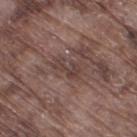Captured during whole-body skin photography for melanoma surveillance; the lesion was not biopsied.
A male subject, aged around 75.
The lesion is on the right thigh.
A region of skin cropped from a whole-body photographic capture, roughly 15 mm wide.